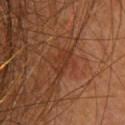The lesion was photographed on a routine skin check and not biopsied; there is no pathology result.
Cropped from a whole-body photographic skin survey; the tile spans about 15 mm.
The recorded lesion diameter is about 4 mm.
The lesion is located on the upper back.
This is a cross-polarized tile.
A female subject approximately 50 years of age.